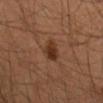Imaged during a routine full-body skin examination; the lesion was not biopsied and no histopathology is available. The lesion is on the right forearm. The patient is a male approximately 35 years of age. A 15 mm close-up tile from a total-body photography series done for melanoma screening.